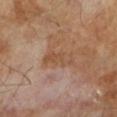Clinical impression:
Imaged during a routine full-body skin examination; the lesion was not biopsied and no histopathology is available.
Background:
Captured under cross-polarized illumination. A roughly 15 mm field-of-view crop from a total-body skin photograph. The subject is a male in their mid- to late 60s.